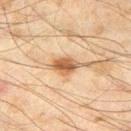notes: no biopsy performed (imaged during a skin exam) | image: ~15 mm tile from a whole-body skin photo | subject: male, aged around 45 | tile lighting: cross-polarized illumination | body site: the right thigh.